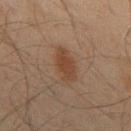Q: Was this lesion biopsied?
A: no biopsy performed (imaged during a skin exam)
Q: How was the tile lit?
A: cross-polarized
Q: Who is the patient?
A: male, about 65 years old
Q: Lesion size?
A: ≈4.5 mm
Q: Lesion location?
A: the mid back
Q: How was this image acquired?
A: 15 mm crop, total-body photography